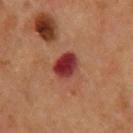{
  "site": "upper back",
  "patient": {
    "sex": "male",
    "age_approx": 60
  },
  "image": {
    "source": "total-body photography crop",
    "field_of_view_mm": 15
  }
}